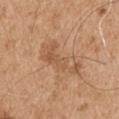Captured during whole-body skin photography for melanoma surveillance; the lesion was not biopsied.
A 15 mm close-up extracted from a 3D total-body photography capture.
The recorded lesion diameter is about 6 mm.
A male patient, aged approximately 65.
The lesion is on the arm.